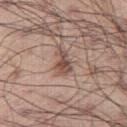Findings:
- follow-up · total-body-photography surveillance lesion; no biopsy
- subject · male, roughly 55 years of age
- lighting · white-light
- location · the leg
- image · 15 mm crop, total-body photography
- image-analysis metrics · a lesion area of about 5 mm², an outline eccentricity of about 0.8 (0 = round, 1 = elongated), and a symmetry-axis asymmetry near 0.3; a lesion–skin lightness drop of about 12 and a normalized lesion–skin contrast near 8.5; a border-irregularity rating of about 3.5/10, a within-lesion color-variation index near 3.5/10, and radial color variation of about 1
- lesion diameter · ~3 mm (longest diameter)